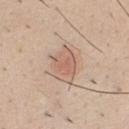Assessment:
Recorded during total-body skin imaging; not selected for excision or biopsy.
Clinical summary:
The patient is a male about 35 years old. The tile uses white-light illumination. Located on the chest. A close-up tile cropped from a whole-body skin photograph, about 15 mm across.